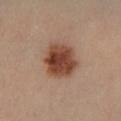- biopsy status — imaged on a skin check; not biopsied
- imaging modality — total-body-photography crop, ~15 mm field of view
- anatomic site — the leg
- tile lighting — cross-polarized illumination
- patient — male, about 40 years old